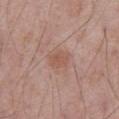The lesion was tiled from a total-body skin photograph and was not biopsied.
A 15 mm crop from a total-body photograph taken for skin-cancer surveillance.
A male patient, in their 70s.
On the front of the torso.